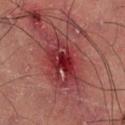Q: Is there a histopathology result?
A: imaged on a skin check; not biopsied
Q: Patient demographics?
A: male, roughly 60 years of age
Q: What is the lesion's diameter?
A: ≈6 mm
Q: What is the imaging modality?
A: total-body-photography crop, ~15 mm field of view